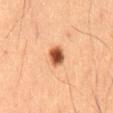Q: Was a biopsy performed?
A: no biopsy performed (imaged during a skin exam)
Q: What is the anatomic site?
A: the mid back
Q: What are the patient's age and sex?
A: male, roughly 65 years of age
Q: What did automated image analysis measure?
A: a lesion area of about 5 mm² and a shape-asymmetry score of about 0.15 (0 = symmetric)
Q: How large is the lesion?
A: ~2.5 mm (longest diameter)
Q: What is the imaging modality?
A: 15 mm crop, total-body photography
Q: How was the tile lit?
A: cross-polarized